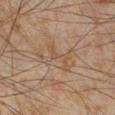This lesion was catalogued during total-body skin photography and was not selected for biopsy.
An algorithmic analysis of the crop reported an area of roughly 8 mm², an outline eccentricity of about 0.85 (0 = round, 1 = elongated), and two-axis asymmetry of about 0.55. The analysis additionally found an average lesion color of about L≈41 a*≈13 b*≈25 (CIELAB). And it measured a color-variation rating of about 1/10.
A lesion tile, about 15 mm wide, cut from a 3D total-body photograph.
From the leg.
The tile uses cross-polarized illumination.
The lesion's longest dimension is about 5.5 mm.
A male patient roughly 45 years of age.